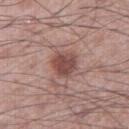Assessment: Part of a total-body skin-imaging series; this lesion was reviewed on a skin check and was not flagged for biopsy. Acquisition and patient details: Longest diameter approximately 3 mm. The tile uses white-light illumination. Automated tile analysis of the lesion measured a lesion–skin lightness drop of about 12 and a lesion-to-skin contrast of about 8.5 (normalized; higher = more distinct). And it measured a lesion-detection confidence of about 100/100. The subject is a male aged 58–62. A roughly 15 mm field-of-view crop from a total-body skin photograph. From the right thigh.